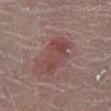* notes: no biopsy performed (imaged during a skin exam)
* patient: female, aged 38 to 42
* acquisition: ~15 mm tile from a whole-body skin photo
* automated lesion analysis: a lesion color around L≈45 a*≈22 b*≈21 in CIELAB and roughly 8 lightness units darker than nearby skin; internal color variation of about 4 on a 0–10 scale and radial color variation of about 1; a classifier nevus-likeness of about 75/100
* site: the leg